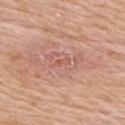image source: ~15 mm tile from a whole-body skin photo | body site: the right upper arm | illumination: white-light | image-analysis metrics: a lesion area of about 1.5 mm², an outline eccentricity of about 0.9 (0 = round, 1 = elongated), and a shape-asymmetry score of about 0.3 (0 = symmetric); a mean CIELAB color near L≈57 a*≈25 b*≈29, a lesion–skin lightness drop of about 6, and a normalized lesion–skin contrast near 4.5; a border-irregularity rating of about 3/10, a within-lesion color-variation index near 0/10, and peripheral color asymmetry of about 0; a lesion-detection confidence of about 95/100 | patient: male, aged around 75 | biopsy diagnosis: a nodular basal cell carcinoma — a skin cancer.